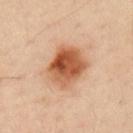Notes:
• follow-up · total-body-photography surveillance lesion; no biopsy
• tile lighting · cross-polarized illumination
• size · ≈5 mm
• acquisition · ~15 mm tile from a whole-body skin photo
• patient · male, aged 48–52
• automated metrics · a lesion area of about 17 mm², an eccentricity of roughly 0.55, and a shape-asymmetry score of about 0.15 (0 = symmetric); a mean CIELAB color near L≈55 a*≈25 b*≈36 and roughly 15 lightness units darker than nearby skin; a border-irregularity rating of about 1.5/10, internal color variation of about 8.5 on a 0–10 scale, and radial color variation of about 3
• body site · the left upper arm